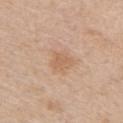{"biopsy_status": "not biopsied; imaged during a skin examination", "patient": {"sex": "male", "age_approx": 60}, "image": {"source": "total-body photography crop", "field_of_view_mm": 15}, "site": "right upper arm"}